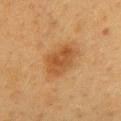The lesion was tiled from a total-body skin photograph and was not biopsied. A female patient, roughly 40 years of age. The lesion is located on the chest. A lesion tile, about 15 mm wide, cut from a 3D total-body photograph. This is a cross-polarized tile.